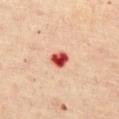  biopsy_status: not biopsied; imaged during a skin examination
  lighting: cross-polarized
  lesion_size:
    long_diameter_mm_approx: 2.5
  patient:
    sex: female
    age_approx: 45
  site: chest
  image:
    source: total-body photography crop
    field_of_view_mm: 15